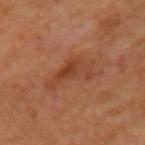Q: Was a biopsy performed?
A: no biopsy performed (imaged during a skin exam)
Q: Who is the patient?
A: female, aged 53–57
Q: What kind of image is this?
A: ~15 mm crop, total-body skin-cancer survey
Q: Lesion location?
A: the arm